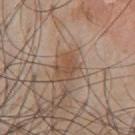Recorded during total-body skin imaging; not selected for excision or biopsy.
A 15 mm close-up tile from a total-body photography series done for melanoma screening.
Captured under white-light illumination.
A male subject aged approximately 60.
The total-body-photography lesion software estimated an average lesion color of about L≈50 a*≈18 b*≈30 (CIELAB), roughly 8 lightness units darker than nearby skin, and a normalized border contrast of about 7. It also reported a border-irregularity index near 6.5/10, internal color variation of about 0.5 on a 0–10 scale, and peripheral color asymmetry of about 0. The analysis additionally found a detector confidence of about 100 out of 100 that the crop contains a lesion.
The lesion is on the chest.